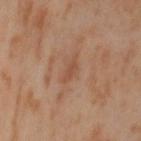image: 15 mm crop, total-body photography
anatomic site: the left thigh
image-analysis metrics: roughly 6 lightness units darker than nearby skin and a lesion-to-skin contrast of about 5 (normalized; higher = more distinct); internal color variation of about 0 on a 0–10 scale and a peripheral color-asymmetry measure near 0
subject: female, in their mid- to late 50s
tile lighting: cross-polarized illumination
lesion diameter: about 3 mm